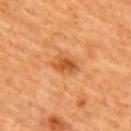Notes:
• biopsy status · total-body-photography surveillance lesion; no biopsy
• patient · female, aged approximately 45
• lighting · cross-polarized
• body site · the upper back
• size · ≈3 mm
• image source · 15 mm crop, total-body photography